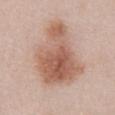workup=no biopsy performed (imaged during a skin exam) | acquisition=~15 mm tile from a whole-body skin photo | diameter=~8 mm (longest diameter) | illumination=white-light illumination | patient=male, aged around 55 | location=the abdomen.